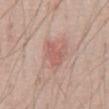Case summary:
• workup · catalogued during a skin exam; not biopsied
• anatomic site · the abdomen
• acquisition · 15 mm crop, total-body photography
• lighting · white-light illumination
• automated lesion analysis · an area of roughly 5 mm² and two-axis asymmetry of about 0.35; a lesion-detection confidence of about 100/100
• subject · male, about 70 years old